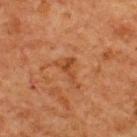No biopsy was performed on this lesion — it was imaged during a full skin examination and was not determined to be concerning.
The lesion is on the upper back.
A male subject in their mid-60s.
A 15 mm close-up tile from a total-body photography series done for melanoma screening.